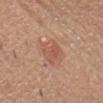Part of a total-body skin-imaging series; this lesion was reviewed on a skin check and was not flagged for biopsy. A 15 mm close-up tile from a total-body photography series done for melanoma screening. From the head or neck. The subject is a male in their 60s. The recorded lesion diameter is about 4 mm. Automated tile analysis of the lesion measured border irregularity of about 2.5 on a 0–10 scale, internal color variation of about 3 on a 0–10 scale, and radial color variation of about 1.